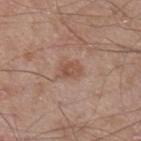Notes:
- follow-up · total-body-photography surveillance lesion; no biopsy
- lesion diameter · about 2.5 mm
- site · the left upper arm
- patient · male, about 65 years old
- tile lighting · white-light illumination
- acquisition · total-body-photography crop, ~15 mm field of view